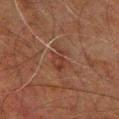Clinical impression: Captured during whole-body skin photography for melanoma surveillance; the lesion was not biopsied. Acquisition and patient details: A lesion tile, about 15 mm wide, cut from a 3D total-body photograph. On the chest. About 3.5 mm across. The subject is a male about 60 years old. Automated image analysis of the tile measured a shape eccentricity near 0.8 and a symmetry-axis asymmetry near 0.3. The software also gave a nevus-likeness score of about 0/100.